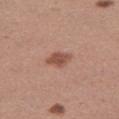This lesion was catalogued during total-body skin photography and was not selected for biopsy.